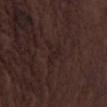The lesion was photographed on a routine skin check and not biopsied; there is no pathology result. From the left thigh. Longest diameter approximately 3 mm. This is a white-light tile. A 15 mm crop from a total-body photograph taken for skin-cancer surveillance. The patient is a male roughly 75 years of age.